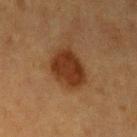Captured during whole-body skin photography for melanoma surveillance; the lesion was not biopsied. Longest diameter approximately 4.5 mm. From the left upper arm. Captured under cross-polarized illumination. A region of skin cropped from a whole-body photographic capture, roughly 15 mm wide. The subject is a female in their mid-50s.